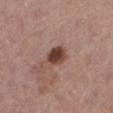{
  "biopsy_status": "not biopsied; imaged during a skin examination",
  "patient": {
    "sex": "female",
    "age_approx": 65
  },
  "lighting": "white-light",
  "site": "left lower leg",
  "image": {
    "source": "total-body photography crop",
    "field_of_view_mm": 15
  }
}